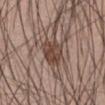Q: Was a biopsy performed?
A: total-body-photography surveillance lesion; no biopsy
Q: What kind of image is this?
A: ~15 mm crop, total-body skin-cancer survey
Q: Illumination type?
A: white-light illumination
Q: What is the anatomic site?
A: the abdomen
Q: How large is the lesion?
A: ~3.5 mm (longest diameter)
Q: Who is the patient?
A: male, aged 63–67
Q: Automated lesion metrics?
A: an average lesion color of about L≈44 a*≈17 b*≈24 (CIELAB), a lesion–skin lightness drop of about 11, and a normalized lesion–skin contrast near 8.5; a peripheral color-asymmetry measure near 1.5